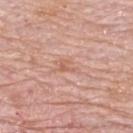Part of a total-body skin-imaging series; this lesion was reviewed on a skin check and was not flagged for biopsy. The patient is a female about 65 years old. On the upper back. The lesion-visualizer software estimated an area of roughly 3.5 mm², an outline eccentricity of about 0.85 (0 = round, 1 = elongated), and a symmetry-axis asymmetry near 0.45. The software also gave an average lesion color of about L≈61 a*≈23 b*≈29 (CIELAB), roughly 7 lightness units darker than nearby skin, and a lesion-to-skin contrast of about 5 (normalized; higher = more distinct). The analysis additionally found a classifier nevus-likeness of about 0/100 and a detector confidence of about 100 out of 100 that the crop contains a lesion. A 15 mm close-up extracted from a 3D total-body photography capture. The tile uses white-light illumination. About 3 mm across.